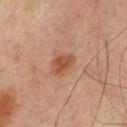Imaged during a routine full-body skin examination; the lesion was not biopsied and no histopathology is available. This is a cross-polarized tile. A male subject approximately 65 years of age. The lesion is on the back. Measured at roughly 3 mm in maximum diameter. A 15 mm crop from a total-body photograph taken for skin-cancer surveillance.